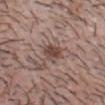follow-up — catalogued during a skin exam; not biopsied
automated lesion analysis — a footprint of about 5 mm², an eccentricity of roughly 0.65, and a symmetry-axis asymmetry near 0.2; about 11 CIELAB-L* units darker than the surrounding skin
site — the head or neck
lighting — white-light illumination
subject — male, in their mid- to late 30s
image — ~15 mm tile from a whole-body skin photo